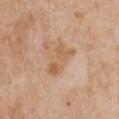biopsy_status: not biopsied; imaged during a skin examination
automated_metrics:
  vs_skin_darker_L: 8.0
  border_irregularity_0_10: 5.5
  color_variation_0_10: 2.5
  peripheral_color_asymmetry: 0.5
  nevus_likeness_0_100: 0
  lesion_detection_confidence_0_100: 100
image:
  source: total-body photography crop
  field_of_view_mm: 15
patient:
  sex: female
  age_approx: 70
site: chest
lighting: white-light